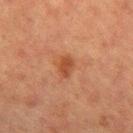<case>
<biopsy_status>not biopsied; imaged during a skin examination</biopsy_status>
<image>
  <source>total-body photography crop</source>
  <field_of_view_mm>15</field_of_view_mm>
</image>
<site>right upper arm</site>
<automated_metrics>
  <area_mm2_approx>3.0</area_mm2_approx>
  <eccentricity>0.85</eccentricity>
  <nevus_likeness_0_100>45</nevus_likeness_0_100>
  <lesion_detection_confidence_0_100>100</lesion_detection_confidence_0_100>
</automated_metrics>
<lighting>cross-polarized</lighting>
<lesion_size>
  <long_diameter_mm_approx>2.5</long_diameter_mm_approx>
</lesion_size>
<patient>
  <sex>female</sex>
  <age_approx>55</age_approx>
</patient>
</case>